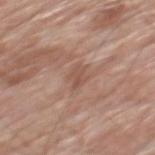Findings:
– workup — catalogued during a skin exam; not biopsied
– imaging modality — total-body-photography crop, ~15 mm field of view
– body site — the mid back
– patient — male, in their 80s